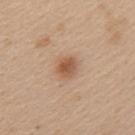| key | value |
|---|---|
| notes | total-body-photography surveillance lesion; no biopsy |
| body site | the left upper arm |
| lighting | white-light |
| image source | ~15 mm tile from a whole-body skin photo |
| lesion size | ~2.5 mm (longest diameter) |
| subject | female, aged around 25 |
| TBP lesion metrics | a symmetry-axis asymmetry near 0.2; an automated nevus-likeness rating near 95 out of 100 and a detector confidence of about 100 out of 100 that the crop contains a lesion |